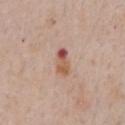• illumination: white-light illumination
• patient: male, aged 58–62
• image-analysis metrics: a footprint of about 4.5 mm² and a shape-asymmetry score of about 0.2 (0 = symmetric); an average lesion color of about L≈55 a*≈23 b*≈29 (CIELAB) and a normalized border contrast of about 8.5; a classifier nevus-likeness of about 0/100 and a detector confidence of about 100 out of 100 that the crop contains a lesion
• diameter: ~3.5 mm (longest diameter)
• imaging modality: total-body-photography crop, ~15 mm field of view
• site: the front of the torso